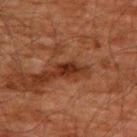No biopsy was performed on this lesion — it was imaged during a full skin examination and was not determined to be concerning.
The subject is a male aged 58–62.
This image is a 15 mm lesion crop taken from a total-body photograph.
The lesion is on the upper back.
Imaged with cross-polarized lighting.
About 2 mm across.